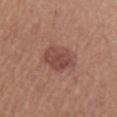notes: imaged on a skin check; not biopsied | image: ~15 mm tile from a whole-body skin photo | body site: the left upper arm | patient: male, in their mid-50s.